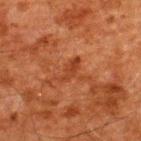notes — catalogued during a skin exam; not biopsied
lighting — cross-polarized illumination
acquisition — ~15 mm tile from a whole-body skin photo
body site — the upper back
diameter — about 3 mm
patient — male, roughly 60 years of age